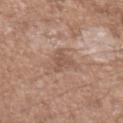Impression: Imaged during a routine full-body skin examination; the lesion was not biopsied and no histopathology is available. Context: A male subject approximately 50 years of age. This is a white-light tile. Approximately 3 mm at its widest. A lesion tile, about 15 mm wide, cut from a 3D total-body photograph. The lesion is on the left forearm.